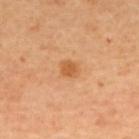Recorded during total-body skin imaging; not selected for excision or biopsy.
Automated tile analysis of the lesion measured an average lesion color of about L≈60 a*≈26 b*≈44 (CIELAB), about 10 CIELAB-L* units darker than the surrounding skin, and a normalized border contrast of about 7.
A female patient in their 50s.
A region of skin cropped from a whole-body photographic capture, roughly 15 mm wide.
Longest diameter approximately 2 mm.
Located on the upper back.
Captured under cross-polarized illumination.